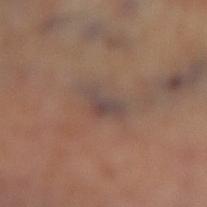{
  "biopsy_status": "not biopsied; imaged during a skin examination",
  "site": "right lower leg",
  "image": {
    "source": "total-body photography crop",
    "field_of_view_mm": 15
  },
  "automated_metrics": {
    "border_irregularity_0_10": 5.0,
    "color_variation_0_10": 3.5
  }
}